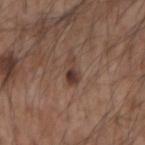Imaged during a routine full-body skin examination; the lesion was not biopsied and no histopathology is available. A male subject aged 53 to 57. A region of skin cropped from a whole-body photographic capture, roughly 15 mm wide. The lesion is on the left forearm.